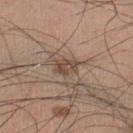biopsy_status: not biopsied; imaged during a skin examination
lighting: white-light
lesion_size:
  long_diameter_mm_approx: 3.0
site: left lower leg
image:
  source: total-body photography crop
  field_of_view_mm: 15
patient:
  sex: male
  age_approx: 35
automated_metrics:
  area_mm2_approx: 4.0
  eccentricity: 0.8
  shape_asymmetry: 0.25
  lesion_detection_confidence_0_100: 95